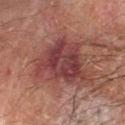biopsy_status: not biopsied; imaged during a skin examination
automated_metrics:
  cielab_L: 39
  cielab_a: 25
  cielab_b: 21
  vs_skin_darker_L: 11.0
  vs_skin_contrast_norm: 9.5
site: right forearm
lighting: cross-polarized
lesion_size:
  long_diameter_mm_approx: 6.5
image:
  source: total-body photography crop
  field_of_view_mm: 15
patient:
  sex: male
  age_approx: 65